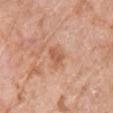<tbp_lesion>
  <biopsy_status>not biopsied; imaged during a skin examination</biopsy_status>
  <automated_metrics>
    <eccentricity>0.6</eccentricity>
    <shape_asymmetry>0.3</shape_asymmetry>
    <cielab_L>59</cielab_L>
    <cielab_a>24</cielab_a>
    <cielab_b>34</cielab_b>
    <vs_skin_darker_L>9.0</vs_skin_darker_L>
    <vs_skin_contrast_norm>6.5</vs_skin_contrast_norm>
    <border_irregularity_0_10>3.0</border_irregularity_0_10>
    <color_variation_0_10>3.0</color_variation_0_10>
    <peripheral_color_asymmetry>1.0</peripheral_color_asymmetry>
  </automated_metrics>
  <lesion_size>
    <long_diameter_mm_approx>3.0</long_diameter_mm_approx>
  </lesion_size>
  <site>front of the torso</site>
  <image>
    <source>total-body photography crop</source>
    <field_of_view_mm>15</field_of_view_mm>
  </image>
  <patient>
    <sex>female</sex>
    <age_approx>75</age_approx>
  </patient>
  <lighting>white-light</lighting>
</tbp_lesion>